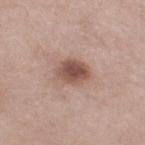| key | value |
|---|---|
| location | the leg |
| imaging modality | ~15 mm tile from a whole-body skin photo |
| subject | female, aged 53 to 57 |
| lesion diameter | ~4 mm (longest diameter) |
| lighting | white-light illumination |
| automated lesion analysis | an outline eccentricity of about 0.7 (0 = round, 1 = elongated) and a shape-asymmetry score of about 0.15 (0 = symmetric); a nevus-likeness score of about 85/100 and a lesion-detection confidence of about 100/100 |